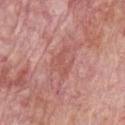  biopsy_status: not biopsied; imaged during a skin examination
  image:
    source: total-body photography crop
    field_of_view_mm: 15
  patient:
    sex: male
    age_approx: 65
  lesion_size:
    long_diameter_mm_approx: 3.5
  site: chest